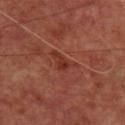Clinical impression: The lesion was photographed on a routine skin check and not biopsied; there is no pathology result. Clinical summary: Measured at roughly 3.5 mm in maximum diameter. The subject is a female aged around 45. A roughly 15 mm field-of-view crop from a total-body skin photograph. Located on the back.